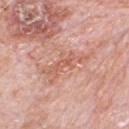biopsy status: total-body-photography surveillance lesion; no biopsy | subject: male, aged 78–82 | location: the chest | size: about 5.5 mm | lighting: white-light | imaging modality: 15 mm crop, total-body photography.